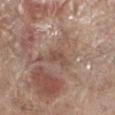Part of a total-body skin-imaging series; this lesion was reviewed on a skin check and was not flagged for biopsy.
The lesion's longest dimension is about 2.5 mm.
The lesion is on the left lower leg.
A roughly 15 mm field-of-view crop from a total-body skin photograph.
The subject is a male in their 70s.
The lesion-visualizer software estimated about 7 CIELAB-L* units darker than the surrounding skin and a normalized lesion–skin contrast near 5.5. And it measured a border-irregularity rating of about 4.5/10 and a color-variation rating of about 1.5/10. The analysis additionally found a nevus-likeness score of about 0/100 and a lesion-detection confidence of about 100/100.
Captured under white-light illumination.